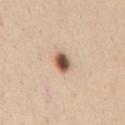Q: Is there a histopathology result?
A: total-body-photography surveillance lesion; no biopsy
Q: How was the tile lit?
A: white-light illumination
Q: Who is the patient?
A: male, aged 28 to 32
Q: What is the lesion's diameter?
A: ≈2.5 mm
Q: Lesion location?
A: the chest
Q: What kind of image is this?
A: ~15 mm tile from a whole-body skin photo